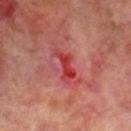This lesion was catalogued during total-body skin photography and was not selected for biopsy.
Measured at roughly 4 mm in maximum diameter.
The lesion is located on the right lower leg.
A close-up tile cropped from a whole-body skin photograph, about 15 mm across.
Captured under cross-polarized illumination.
Automated tile analysis of the lesion measured a footprint of about 6 mm², an eccentricity of roughly 0.9, and a symmetry-axis asymmetry near 0.55. And it measured a lesion color around L≈43 a*≈42 b*≈29 in CIELAB and roughly 10 lightness units darker than nearby skin. It also reported a border-irregularity rating of about 6.5/10 and a color-variation rating of about 4/10. And it measured a nevus-likeness score of about 0/100 and a lesion-detection confidence of about 55/100.
The patient is a male aged around 70.